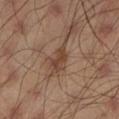  biopsy_status: not biopsied; imaged during a skin examination
  automated_metrics:
    nevus_likeness_0_100: 20
    lesion_detection_confidence_0_100: 100
  lesion_size:
    long_diameter_mm_approx: 3.0
  lighting: cross-polarized
  image:
    source: total-body photography crop
    field_of_view_mm: 15
  patient:
    sex: male
    age_approx: 45
  site: left lower leg